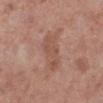{"image": {"source": "total-body photography crop", "field_of_view_mm": 15}, "patient": {"sex": "female", "age_approx": 50}, "lesion_size": {"long_diameter_mm_approx": 5.0}, "lighting": "white-light", "site": "left lower leg"}